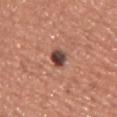The lesion was photographed on a routine skin check and not biopsied; there is no pathology result.
Measured at roughly 2.5 mm in maximum diameter.
A 15 mm crop from a total-body photograph taken for skin-cancer surveillance.
Automated image analysis of the tile measured a shape eccentricity near 0.65 and a symmetry-axis asymmetry near 0.15.
A male subject, roughly 45 years of age.
On the chest.